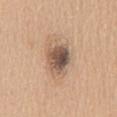Q: Is there a histopathology result?
A: catalogued during a skin exam; not biopsied
Q: Lesion location?
A: the back
Q: Who is the patient?
A: female, aged 68 to 72
Q: What did automated image analysis measure?
A: a classifier nevus-likeness of about 5/100 and a detector confidence of about 100 out of 100 that the crop contains a lesion
Q: How was this image acquired?
A: total-body-photography crop, ~15 mm field of view
Q: What lighting was used for the tile?
A: white-light illumination